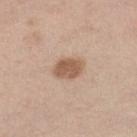patient = female, aged around 55
anatomic site = the left lower leg
image source = total-body-photography crop, ~15 mm field of view